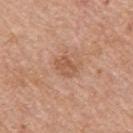The lesion was tiled from a total-body skin photograph and was not biopsied.
Longest diameter approximately 2.5 mm.
This image is a 15 mm lesion crop taken from a total-body photograph.
An algorithmic analysis of the crop reported about 8 CIELAB-L* units darker than the surrounding skin and a normalized lesion–skin contrast near 6. It also reported a border-irregularity index near 2.5/10, internal color variation of about 2.5 on a 0–10 scale, and radial color variation of about 1. And it measured a detector confidence of about 100 out of 100 that the crop contains a lesion.
On the upper back.
This is a white-light tile.
A male patient, aged around 60.